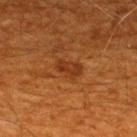The lesion was photographed on a routine skin check and not biopsied; there is no pathology result. A lesion tile, about 15 mm wide, cut from a 3D total-body photograph. This is a cross-polarized tile. From the upper back. The patient is a male aged 58–62. Automated tile analysis of the lesion measured a border-irregularity rating of about 2/10, a color-variation rating of about 2.5/10, and a peripheral color-asymmetry measure near 1. Approximately 3 mm at its widest.